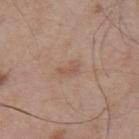Assessment: The lesion was tiled from a total-body skin photograph and was not biopsied. Image and clinical context: Cropped from a whole-body photographic skin survey; the tile spans about 15 mm. This is a white-light tile. The recorded lesion diameter is about 3 mm. Automated tile analysis of the lesion measured an eccentricity of roughly 0.85 and a symmetry-axis asymmetry near 0.4. And it measured lesion-presence confidence of about 100/100. A male subject aged 53 to 57. The lesion is on the mid back.